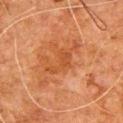Recorded during total-body skin imaging; not selected for excision or biopsy.
A male patient, approximately 80 years of age.
Located on the chest.
Automated image analysis of the tile measured a footprint of about 6 mm² and an outline eccentricity of about 0.85 (0 = round, 1 = elongated). It also reported a mean CIELAB color near L≈40 a*≈24 b*≈35, roughly 5 lightness units darker than nearby skin, and a lesion-to-skin contrast of about 5 (normalized; higher = more distinct). And it measured a lesion-detection confidence of about 100/100.
The tile uses cross-polarized illumination.
A 15 mm close-up extracted from a 3D total-body photography capture.
Longest diameter approximately 4 mm.